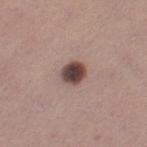Assessment:
This lesion was catalogued during total-body skin photography and was not selected for biopsy.
Clinical summary:
The lesion is located on the left thigh. A female patient aged 28–32. Approximately 3 mm at its widest. Imaged with white-light lighting. A region of skin cropped from a whole-body photographic capture, roughly 15 mm wide.